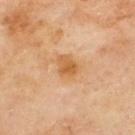Impression: Part of a total-body skin-imaging series; this lesion was reviewed on a skin check and was not flagged for biopsy. Clinical summary: Measured at roughly 3.5 mm in maximum diameter. The lesion is located on the upper back. A male subject aged approximately 70. A 15 mm close-up tile from a total-body photography series done for melanoma screening.